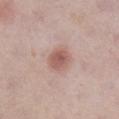A female subject aged 58 to 62. From the right lower leg. The lesion's longest dimension is about 3 mm. A close-up tile cropped from a whole-body skin photograph, about 15 mm across. Imaged with white-light lighting. Automated image analysis of the tile measured a footprint of about 6.5 mm² and a shape-asymmetry score of about 0.15 (0 = symmetric). The software also gave a nevus-likeness score of about 90/100.